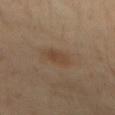Q: Was a biopsy performed?
A: no biopsy performed (imaged during a skin exam)
Q: How was the tile lit?
A: cross-polarized
Q: How was this image acquired?
A: ~15 mm tile from a whole-body skin photo
Q: Where on the body is the lesion?
A: the mid back
Q: How large is the lesion?
A: ~3 mm (longest diameter)
Q: Who is the patient?
A: male, aged 28–32